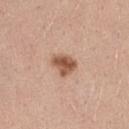Part of a total-body skin-imaging series; this lesion was reviewed on a skin check and was not flagged for biopsy. Automated image analysis of the tile measured a lesion area of about 6.5 mm², an outline eccentricity of about 0.6 (0 = round, 1 = elongated), and two-axis asymmetry of about 0.25. It also reported a lesion–skin lightness drop of about 14 and a lesion-to-skin contrast of about 9.5 (normalized; higher = more distinct). The software also gave border irregularity of about 2.5 on a 0–10 scale, a color-variation rating of about 4/10, and a peripheral color-asymmetry measure near 1.5. Cropped from a total-body skin-imaging series; the visible field is about 15 mm. The lesion's longest dimension is about 3 mm. This is a white-light tile. A female subject aged around 45. The lesion is located on the left thigh.